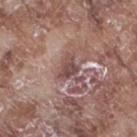The lesion was tiled from a total-body skin photograph and was not biopsied. Approximately 2.5 mm at its widest. An algorithmic analysis of the crop reported an outline eccentricity of about 0.6 (0 = round, 1 = elongated). A 15 mm close-up tile from a total-body photography series done for melanoma screening. This is a white-light tile. On the right thigh. A male subject, aged 73–77.